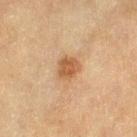The lesion was photographed on a routine skin check and not biopsied; there is no pathology result. Cropped from a whole-body photographic skin survey; the tile spans about 15 mm. The lesion is on the right thigh. A female patient, roughly 80 years of age.